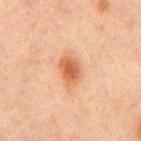Captured during whole-body skin photography for melanoma surveillance; the lesion was not biopsied. This image is a 15 mm lesion crop taken from a total-body photograph. From the mid back. A male subject aged approximately 65.